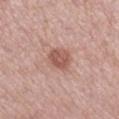Assessment:
Recorded during total-body skin imaging; not selected for excision or biopsy.
Background:
A 15 mm close-up extracted from a 3D total-body photography capture. A female subject, about 55 years old. Captured under white-light illumination. Approximately 3 mm at its widest. Located on the right lower leg.